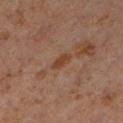biopsy_status: not biopsied; imaged during a skin examination
lighting: cross-polarized
patient:
  sex: male
  age_approx: 60
lesion_size:
  long_diameter_mm_approx: 2.5
image:
  source: total-body photography crop
  field_of_view_mm: 15
site: left lower leg